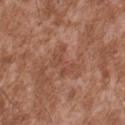Impression: The lesion was tiled from a total-body skin photograph and was not biopsied. Acquisition and patient details: A roughly 15 mm field-of-view crop from a total-body skin photograph. The tile uses white-light illumination. From the upper back. The lesion's longest dimension is about 3 mm. A male subject, aged approximately 45. Automated image analysis of the tile measured a mean CIELAB color near L≈47 a*≈23 b*≈29, a lesion–skin lightness drop of about 6, and a normalized lesion–skin contrast near 5. The analysis additionally found a color-variation rating of about 1.5/10 and radial color variation of about 0.5.